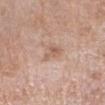<record>
<biopsy_status>not biopsied; imaged during a skin examination</biopsy_status>
<site>right forearm</site>
<patient>
  <sex>female</sex>
  <age_approx>55</age_approx>
</patient>
<lighting>white-light</lighting>
<lesion_size>
  <long_diameter_mm_approx>3.0</long_diameter_mm_approx>
</lesion_size>
<image>
  <source>total-body photography crop</source>
  <field_of_view_mm>15</field_of_view_mm>
</image>
</record>